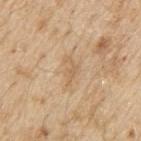Part of a total-body skin-imaging series; this lesion was reviewed on a skin check and was not flagged for biopsy. Automated tile analysis of the lesion measured a classifier nevus-likeness of about 0/100 and a detector confidence of about 100 out of 100 that the crop contains a lesion. Longest diameter approximately 3.5 mm. The lesion is on the right upper arm. A 15 mm close-up tile from a total-body photography series done for melanoma screening. The subject is a male aged approximately 70.